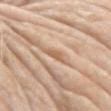This lesion was catalogued during total-body skin photography and was not selected for biopsy.
Located on the left upper arm.
The lesion's longest dimension is about 3 mm.
A male subject, in their mid- to late 70s.
A 15 mm close-up tile from a total-body photography series done for melanoma screening.